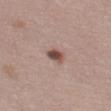No biopsy was performed on this lesion — it was imaged during a full skin examination and was not determined to be concerning.
A roughly 15 mm field-of-view crop from a total-body skin photograph.
The tile uses white-light illumination.
The recorded lesion diameter is about 2.5 mm.
A female subject aged approximately 40.
Automated image analysis of the tile measured a border-irregularity rating of about 2.5/10, a color-variation rating of about 6/10, and a peripheral color-asymmetry measure near 2. The analysis additionally found a lesion-detection confidence of about 100/100.
On the left thigh.